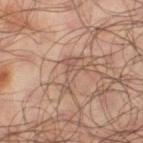Q: How large is the lesion?
A: ~4 mm (longest diameter)
Q: What lighting was used for the tile?
A: cross-polarized
Q: Who is the patient?
A: male, aged 63 to 67
Q: Lesion location?
A: the left thigh
Q: What did automated image analysis measure?
A: an area of roughly 5.5 mm², an outline eccentricity of about 0.9 (0 = round, 1 = elongated), and a symmetry-axis asymmetry near 0.6; a lesion color around L≈50 a*≈18 b*≈25 in CIELAB, a lesion–skin lightness drop of about 7, and a lesion-to-skin contrast of about 5.5 (normalized; higher = more distinct)
Q: How was this image acquired?
A: ~15 mm tile from a whole-body skin photo